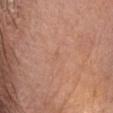Imaged during a routine full-body skin examination; the lesion was not biopsied and no histopathology is available. About 1 mm across. A female subject roughly 50 years of age. The lesion is on the head or neck. A 15 mm close-up extracted from a 3D total-body photography capture.